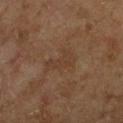Q: Was a biopsy performed?
A: total-body-photography surveillance lesion; no biopsy
Q: Lesion size?
A: ~4 mm (longest diameter)
Q: What are the patient's age and sex?
A: male, aged around 60
Q: Illumination type?
A: cross-polarized illumination
Q: How was this image acquired?
A: ~15 mm tile from a whole-body skin photo
Q: What is the anatomic site?
A: the left lower leg